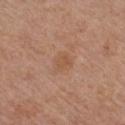No biopsy was performed on this lesion — it was imaged during a full skin examination and was not determined to be concerning. A 15 mm close-up tile from a total-body photography series done for melanoma screening. The patient is a female roughly 55 years of age. The lesion is on the leg.